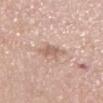Q: Is there a histopathology result?
A: imaged on a skin check; not biopsied
Q: What lighting was used for the tile?
A: white-light illumination
Q: Patient demographics?
A: female, aged 63–67
Q: How large is the lesion?
A: about 3 mm
Q: Where on the body is the lesion?
A: the leg
Q: Automated lesion metrics?
A: a lesion area of about 5.5 mm² and a symmetry-axis asymmetry near 0.3; a mean CIELAB color near L≈62 a*≈19 b*≈27, roughly 9 lightness units darker than nearby skin, and a normalized lesion–skin contrast near 6
Q: How was this image acquired?
A: 15 mm crop, total-body photography